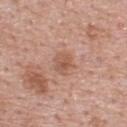Imaged during a routine full-body skin examination; the lesion was not biopsied and no histopathology is available.
A 15 mm crop from a total-body photograph taken for skin-cancer surveillance.
Captured under white-light illumination.
The total-body-photography lesion software estimated a mean CIELAB color near L≈56 a*≈22 b*≈30.
A male patient, aged approximately 55.
On the upper back.